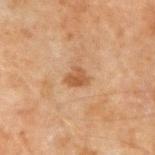A male patient aged around 45.
A roughly 15 mm field-of-view crop from a total-body skin photograph.
Approximately 2.5 mm at its widest.
From the right forearm.
The total-body-photography lesion software estimated a lesion color around L≈45 a*≈19 b*≈31 in CIELAB, a lesion–skin lightness drop of about 8, and a normalized lesion–skin contrast near 7.